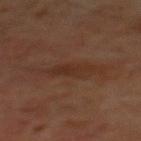biopsy status — catalogued during a skin exam; not biopsied
tile lighting — cross-polarized
image-analysis metrics — a footprint of about 6 mm² and an eccentricity of roughly 0.9
body site — the chest
subject — male, aged 58–62
image source — ~15 mm crop, total-body skin-cancer survey
size — about 4 mm